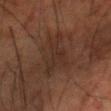Captured during whole-body skin photography for melanoma surveillance; the lesion was not biopsied. Located on the head or neck. The patient is a male roughly 70 years of age. Cropped from a whole-body photographic skin survey; the tile spans about 15 mm.